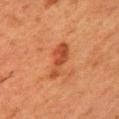notes — total-body-photography surveillance lesion; no biopsy
automated lesion analysis — an area of roughly 6.5 mm²; a classifier nevus-likeness of about 5/100 and lesion-presence confidence of about 100/100
lighting — cross-polarized illumination
subject — female, approximately 50 years of age
anatomic site — the left upper arm
lesion diameter — ≈5 mm
image source — ~15 mm crop, total-body skin-cancer survey